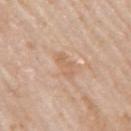Notes:
- workup — catalogued during a skin exam; not biopsied
- illumination — white-light illumination
- automated metrics — a footprint of about 3.5 mm², an outline eccentricity of about 0.9 (0 = round, 1 = elongated), and two-axis asymmetry of about 0.55; a within-lesion color-variation index near 0/10 and radial color variation of about 0; an automated nevus-likeness rating near 0 out of 100 and a lesion-detection confidence of about 100/100
- site — the arm
- subject — male, roughly 75 years of age
- acquisition — total-body-photography crop, ~15 mm field of view
- size — about 3 mm